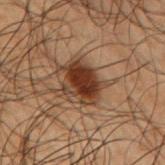notes=catalogued during a skin exam; not biopsied | illumination=cross-polarized illumination | lesion diameter=≈4 mm | imaging modality=15 mm crop, total-body photography | subject=male, aged 48–52 | anatomic site=the right upper arm | TBP lesion metrics=a detector confidence of about 100 out of 100 that the crop contains a lesion.